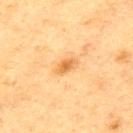follow-up=imaged on a skin check; not biopsied
size=about 2.5 mm
TBP lesion metrics=a footprint of about 3 mm², an eccentricity of roughly 0.85, and two-axis asymmetry of about 0.2; border irregularity of about 2 on a 0–10 scale, internal color variation of about 3 on a 0–10 scale, and a peripheral color-asymmetry measure near 1; a nevus-likeness score of about 50/100
subject=male, roughly 75 years of age
image source=~15 mm crop, total-body skin-cancer survey
tile lighting=cross-polarized illumination
site=the back